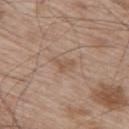image: ~15 mm tile from a whole-body skin photo | site: the upper back | diameter: about 2.5 mm | TBP lesion metrics: a lesion area of about 3.5 mm², a shape eccentricity near 0.75, and two-axis asymmetry of about 0.55; a mean CIELAB color near L≈54 a*≈17 b*≈29, roughly 7 lightness units darker than nearby skin, and a normalized border contrast of about 5.5; a lesion-detection confidence of about 100/100 | patient: male, approximately 65 years of age.